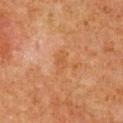Imaged during a routine full-body skin examination; the lesion was not biopsied and no histopathology is available. A region of skin cropped from a whole-body photographic capture, roughly 15 mm wide. Measured at roughly 2.5 mm in maximum diameter. The tile uses cross-polarized illumination. The subject is a male aged approximately 80. An algorithmic analysis of the crop reported a footprint of about 3.5 mm² and an eccentricity of roughly 0.85. The analysis additionally found a lesion color around L≈43 a*≈21 b*≈32 in CIELAB, roughly 5 lightness units darker than nearby skin, and a normalized lesion–skin contrast near 5. The analysis additionally found a border-irregularity index near 2.5/10, a within-lesion color-variation index near 2.5/10, and radial color variation of about 1. The lesion is located on the chest.